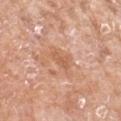Q: Was a biopsy performed?
A: total-body-photography surveillance lesion; no biopsy
Q: What kind of image is this?
A: ~15 mm tile from a whole-body skin photo
Q: Who is the patient?
A: female, in their mid- to late 70s
Q: What is the anatomic site?
A: the arm
Q: What is the lesion's diameter?
A: ≈3 mm
Q: What lighting was used for the tile?
A: white-light